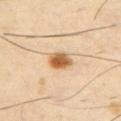This lesion was catalogued during total-body skin photography and was not selected for biopsy. Imaged with cross-polarized lighting. Cropped from a whole-body photographic skin survey; the tile spans about 15 mm. Automated tile analysis of the lesion measured a lesion color around L≈60 a*≈20 b*≈39 in CIELAB, roughly 16 lightness units darker than nearby skin, and a normalized border contrast of about 10.5. The lesion is located on the chest. The lesion's longest dimension is about 3.5 mm. A male patient, aged 38 to 42.